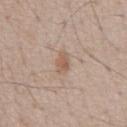| feature | finding |
|---|---|
| subject | male, aged 53–57 |
| diameter | about 2.5 mm |
| automated lesion analysis | an area of roughly 3.5 mm²; a border-irregularity index near 2.5/10, a color-variation rating of about 1.5/10, and radial color variation of about 0.5 |
| imaging modality | total-body-photography crop, ~15 mm field of view |